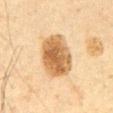Assessment: Recorded during total-body skin imaging; not selected for excision or biopsy. Context: The lesion-visualizer software estimated a lesion area of about 19 mm², an eccentricity of roughly 0.7, and a shape-asymmetry score of about 0.1 (0 = symmetric). And it measured an average lesion color of about L≈53 a*≈16 b*≈35 (CIELAB) and a normalized border contrast of about 10. And it measured a classifier nevus-likeness of about 95/100. Cropped from a whole-body photographic skin survey; the tile spans about 15 mm. Captured under cross-polarized illumination. The lesion is located on the abdomen. The lesion's longest dimension is about 5.5 mm. A male patient in their 60s.